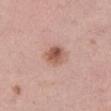The lesion was tiled from a total-body skin photograph and was not biopsied.
The lesion's longest dimension is about 3 mm.
Cropped from a whole-body photographic skin survey; the tile spans about 15 mm.
The lesion is on the leg.
The tile uses white-light illumination.
A female subject, aged 43–47.
An algorithmic analysis of the crop reported a footprint of about 6 mm², an eccentricity of roughly 0.4, and two-axis asymmetry of about 0.15. It also reported an average lesion color of about L≈56 a*≈23 b*≈27 (CIELAB) and a normalized border contrast of about 8.5. And it measured a border-irregularity index near 1.5/10, a within-lesion color-variation index near 5.5/10, and radial color variation of about 2.